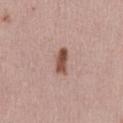• biopsy status: total-body-photography surveillance lesion; no biopsy
• image source: ~15 mm tile from a whole-body skin photo
• lesion size: ~3.5 mm (longest diameter)
• subject: male, roughly 30 years of age